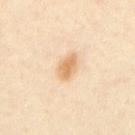No biopsy was performed on this lesion — it was imaged during a full skin examination and was not determined to be concerning. The patient is a male about 30 years old. Approximately 3 mm at its widest. Imaged with cross-polarized lighting. The lesion-visualizer software estimated a footprint of about 5.5 mm² and a shape-asymmetry score of about 0.15 (0 = symmetric). The analysis additionally found a mean CIELAB color near L≈74 a*≈19 b*≈40, a lesion–skin lightness drop of about 11, and a normalized lesion–skin contrast near 8. The software also gave radial color variation of about 1. This image is a 15 mm lesion crop taken from a total-body photograph. The lesion is located on the chest.